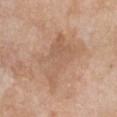Clinical impression: Imaged during a routine full-body skin examination; the lesion was not biopsied and no histopathology is available. Clinical summary: This is a white-light tile. The recorded lesion diameter is about 6.5 mm. Cropped from a whole-body photographic skin survey; the tile spans about 15 mm. From the chest. A female patient, in their mid- to late 60s.